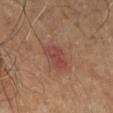Findings:
• biopsy status — total-body-photography surveillance lesion; no biopsy
• location — the lower back
• illumination — cross-polarized
• image — ~15 mm crop, total-body skin-cancer survey
• patient — male, aged 58 to 62